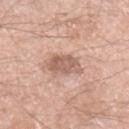Imaged during a routine full-body skin examination; the lesion was not biopsied and no histopathology is available. A male subject aged around 60. On the left upper arm. A close-up tile cropped from a whole-body skin photograph, about 15 mm across. The recorded lesion diameter is about 4 mm.